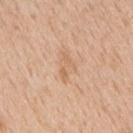<tbp_lesion>
  <biopsy_status>not biopsied; imaged during a skin examination</biopsy_status>
  <image>
    <source>total-body photography crop</source>
    <field_of_view_mm>15</field_of_view_mm>
  </image>
  <patient>
    <sex>male</sex>
    <age_approx>65</age_approx>
  </patient>
  <site>mid back</site>
</tbp_lesion>